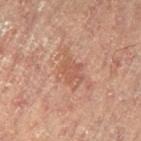- workup — catalogued during a skin exam; not biopsied
- image — total-body-photography crop, ~15 mm field of view
- anatomic site — the right leg
- patient — female, roughly 80 years of age